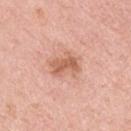Captured during whole-body skin photography for melanoma surveillance; the lesion was not biopsied. Automated image analysis of the tile measured an area of roughly 7.5 mm² and two-axis asymmetry of about 0.25. The software also gave an average lesion color of about L≈62 a*≈24 b*≈32 (CIELAB), about 11 CIELAB-L* units darker than the surrounding skin, and a normalized border contrast of about 7. Located on the left upper arm. Cropped from a whole-body photographic skin survey; the tile spans about 15 mm. A male patient in their 40s. Captured under white-light illumination.